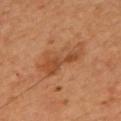Assessment:
Recorded during total-body skin imaging; not selected for excision or biopsy.
Acquisition and patient details:
Automated tile analysis of the lesion measured a lesion area of about 10 mm² and a shape-asymmetry score of about 0.5 (0 = symmetric). And it measured a mean CIELAB color near L≈43 a*≈23 b*≈34, a lesion–skin lightness drop of about 8, and a normalized border contrast of about 6.5. The analysis additionally found a border-irregularity rating of about 7/10, internal color variation of about 2.5 on a 0–10 scale, and a peripheral color-asymmetry measure near 0.5. It also reported an automated nevus-likeness rating near 15 out of 100 and a detector confidence of about 100 out of 100 that the crop contains a lesion. A male patient, approximately 55 years of age. A 15 mm crop from a total-body photograph taken for skin-cancer surveillance. From the back. The recorded lesion diameter is about 6.5 mm. Captured under cross-polarized illumination.